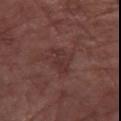Imaged during a routine full-body skin examination; the lesion was not biopsied and no histopathology is available.
The total-body-photography lesion software estimated an average lesion color of about L≈32 a*≈20 b*≈20 (CIELAB), roughly 5 lightness units darker than nearby skin, and a normalized lesion–skin contrast near 5.5. And it measured border irregularity of about 3 on a 0–10 scale, a color-variation rating of about 2/10, and peripheral color asymmetry of about 0.5. The software also gave a classifier nevus-likeness of about 0/100 and lesion-presence confidence of about 100/100.
A lesion tile, about 15 mm wide, cut from a 3D total-body photograph.
On the left forearm.
A female subject approximately 70 years of age.
Longest diameter approximately 4 mm.